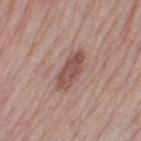Q: Is there a histopathology result?
A: imaged on a skin check; not biopsied
Q: What did automated image analysis measure?
A: a lesion area of about 8 mm², a shape eccentricity near 0.9, and a shape-asymmetry score of about 0.2 (0 = symmetric); a border-irregularity index near 2.5/10, internal color variation of about 3 on a 0–10 scale, and radial color variation of about 1; a nevus-likeness score of about 40/100 and a lesion-detection confidence of about 100/100
Q: What kind of image is this?
A: ~15 mm crop, total-body skin-cancer survey
Q: What are the patient's age and sex?
A: female, aged 63–67
Q: Where on the body is the lesion?
A: the left thigh